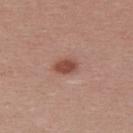The lesion was tiled from a total-body skin photograph and was not biopsied.